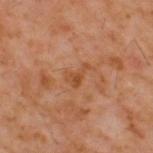The lesion was photographed on a routine skin check and not biopsied; there is no pathology result. From the back. Cropped from a whole-body photographic skin survey; the tile spans about 15 mm. The tile uses cross-polarized illumination. Approximately 3 mm at its widest. A male patient, about 60 years old. Automated tile analysis of the lesion measured a lesion color around L≈45 a*≈23 b*≈35 in CIELAB and a normalized border contrast of about 6.5.